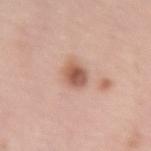This lesion was catalogued during total-body skin photography and was not selected for biopsy. A female subject aged approximately 50. The lesion is on the right upper arm. Cropped from a whole-body photographic skin survey; the tile spans about 15 mm. Imaged with white-light lighting. An algorithmic analysis of the crop reported a lesion color around L≈57 a*≈22 b*≈29 in CIELAB, a lesion–skin lightness drop of about 14, and a normalized lesion–skin contrast near 9. And it measured a border-irregularity rating of about 1.5/10, a within-lesion color-variation index near 6/10, and a peripheral color-asymmetry measure near 2. The recorded lesion diameter is about 3.5 mm.